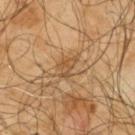Case summary:
* subject — male, about 65 years old
* image source — total-body-photography crop, ~15 mm field of view
* anatomic site — the upper back